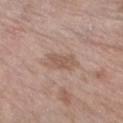The lesion was photographed on a routine skin check and not biopsied; there is no pathology result. A region of skin cropped from a whole-body photographic capture, roughly 15 mm wide. Imaged with white-light lighting. The lesion is on the leg. The lesion's longest dimension is about 3.5 mm. The subject is a female about 85 years old or older.